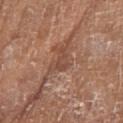Captured during whole-body skin photography for melanoma surveillance; the lesion was not biopsied.
A lesion tile, about 15 mm wide, cut from a 3D total-body photograph.
This is a white-light tile.
The total-body-photography lesion software estimated a shape eccentricity near 0.9 and a symmetry-axis asymmetry near 0.55. The analysis additionally found a mean CIELAB color near L≈46 a*≈22 b*≈28, about 8 CIELAB-L* units darker than the surrounding skin, and a normalized border contrast of about 6.
A female patient aged 73–77.
The lesion is located on the left lower leg.
Longest diameter approximately 3 mm.